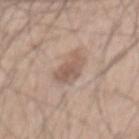  biopsy_status: not biopsied; imaged during a skin examination
  lighting: white-light
  lesion_size:
    long_diameter_mm_approx: 4.0
  patient:
    sex: male
    age_approx: 45
  automated_metrics:
    eccentricity: 0.85
    shape_asymmetry: 0.3
    cielab_L: 56
    cielab_a: 16
    cielab_b: 25
    vs_skin_darker_L: 10.0
    vs_skin_contrast_norm: 6.5
    nevus_likeness_0_100: 20
  image:
    source: total-body photography crop
    field_of_view_mm: 15
  site: mid back